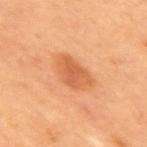The lesion was photographed on a routine skin check and not biopsied; there is no pathology result.
Automated tile analysis of the lesion measured a mean CIELAB color near L≈59 a*≈29 b*≈41, roughly 10 lightness units darker than nearby skin, and a normalized lesion–skin contrast near 6.5. The software also gave border irregularity of about 2 on a 0–10 scale and a within-lesion color-variation index near 3/10.
Cropped from a total-body skin-imaging series; the visible field is about 15 mm.
Captured under cross-polarized illumination.
Longest diameter approximately 4.5 mm.
A female patient, roughly 70 years of age.
The lesion is located on the upper back.